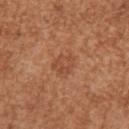Notes:
• notes: total-body-photography surveillance lesion; no biopsy
• image: ~15 mm tile from a whole-body skin photo
• site: the right upper arm
• subject: female, roughly 40 years of age
• lesion diameter: ≈3 mm
• tile lighting: white-light illumination
• automated metrics: a lesion area of about 6.5 mm², an eccentricity of roughly 0.6, and two-axis asymmetry of about 0.25; a lesion-to-skin contrast of about 4.5 (normalized; higher = more distinct); a lesion-detection confidence of about 100/100